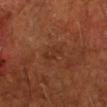The lesion was tiled from a total-body skin photograph and was not biopsied. Imaged with cross-polarized lighting. A roughly 15 mm field-of-view crop from a total-body skin photograph. An algorithmic analysis of the crop reported an outline eccentricity of about 0.9 (0 = round, 1 = elongated) and a shape-asymmetry score of about 0.4 (0 = symmetric). The analysis additionally found a mean CIELAB color near L≈30 a*≈22 b*≈29 and about 5 CIELAB-L* units darker than the surrounding skin. And it measured an automated nevus-likeness rating near 0 out of 100. The recorded lesion diameter is about 3 mm. A male patient, aged 58–62. From the right forearm.